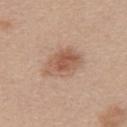The lesion was tiled from a total-body skin photograph and was not biopsied.
On the upper back.
A female patient, approximately 40 years of age.
A region of skin cropped from a whole-body photographic capture, roughly 15 mm wide.
Imaged with white-light lighting.
The total-body-photography lesion software estimated a lesion area of about 14 mm² and a shape eccentricity near 0.75. And it measured an average lesion color of about L≈57 a*≈20 b*≈29 (CIELAB), a lesion–skin lightness drop of about 10, and a lesion-to-skin contrast of about 7 (normalized; higher = more distinct). And it measured a border-irregularity rating of about 2/10, a within-lesion color-variation index near 5/10, and radial color variation of about 1.5. And it measured an automated nevus-likeness rating near 85 out of 100 and a lesion-detection confidence of about 100/100.